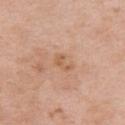Impression: Part of a total-body skin-imaging series; this lesion was reviewed on a skin check and was not flagged for biopsy. Background: A lesion tile, about 15 mm wide, cut from a 3D total-body photograph. Imaged with white-light lighting. Automated image analysis of the tile measured a border-irregularity rating of about 5/10, a color-variation rating of about 1/10, and radial color variation of about 0.5. The analysis additionally found an automated nevus-likeness rating near 0 out of 100 and a detector confidence of about 100 out of 100 that the crop contains a lesion. A female patient aged 48–52. From the front of the torso. Longest diameter approximately 2.5 mm.